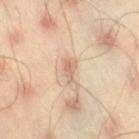| key | value |
|---|---|
| workup | total-body-photography surveillance lesion; no biopsy |
| lighting | cross-polarized |
| site | the right thigh |
| imaging modality | ~15 mm tile from a whole-body skin photo |
| patient | male, aged approximately 45 |